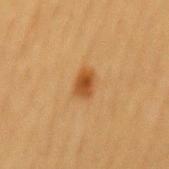Assessment:
The lesion was photographed on a routine skin check and not biopsied; there is no pathology result.
Background:
The lesion is on the mid back. A male subject aged approximately 55. Cropped from a whole-body photographic skin survey; the tile spans about 15 mm.